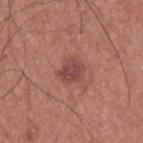Findings:
– biopsy status: total-body-photography surveillance lesion; no biopsy
– diameter: about 3 mm
– location: the back
– acquisition: 15 mm crop, total-body photography
– lighting: white-light
– subject: male, aged around 35